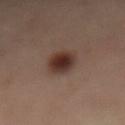A male subject, aged 53–57. The tile uses cross-polarized illumination. On the mid back. A lesion tile, about 15 mm wide, cut from a 3D total-body photograph. The lesion's longest dimension is about 3.5 mm.